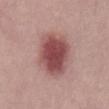follow-up: catalogued during a skin exam; not biopsied
lighting: white-light illumination
automated metrics: a footprint of about 21 mm², a shape eccentricity near 0.7, and a shape-asymmetry score of about 0.15 (0 = symmetric); a lesion color around L≈49 a*≈25 b*≈21 in CIELAB and a normalized lesion–skin contrast near 10.5; an automated nevus-likeness rating near 95 out of 100 and lesion-presence confidence of about 100/100
imaging modality: total-body-photography crop, ~15 mm field of view
subject: male, roughly 30 years of age